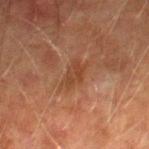Recorded during total-body skin imaging; not selected for excision or biopsy. From the right thigh. A male patient in their mid- to late 70s. An algorithmic analysis of the crop reported a footprint of about 5.5 mm² and two-axis asymmetry of about 0.35. And it measured a border-irregularity rating of about 4.5/10, internal color variation of about 2.5 on a 0–10 scale, and peripheral color asymmetry of about 1. The software also gave a classifier nevus-likeness of about 0/100 and a lesion-detection confidence of about 100/100. Measured at roughly 3.5 mm in maximum diameter. Cropped from a whole-body photographic skin survey; the tile spans about 15 mm.